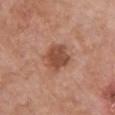follow-up: catalogued during a skin exam; not biopsied | lesion diameter: ~3.5 mm (longest diameter) | image source: total-body-photography crop, ~15 mm field of view | illumination: white-light | patient: female, approximately 60 years of age | site: the front of the torso.